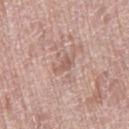Clinical impression: The lesion was tiled from a total-body skin photograph and was not biopsied. Acquisition and patient details: The tile uses white-light illumination. From the right thigh. The recorded lesion diameter is about 2.5 mm. The subject is a female aged around 60. A 15 mm close-up tile from a total-body photography series done for melanoma screening.